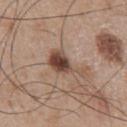biopsy status = total-body-photography surveillance lesion; no biopsy | tile lighting = white-light | location = the chest | imaging modality = ~15 mm tile from a whole-body skin photo | lesion diameter = ~5 mm (longest diameter) | patient = male, approximately 65 years of age.